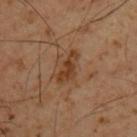Case summary:
- workup: no biopsy performed (imaged during a skin exam)
- diameter: ~4 mm (longest diameter)
- site: the upper back
- subject: male, aged 48 to 52
- imaging modality: ~15 mm tile from a whole-body skin photo
- lighting: cross-polarized illumination